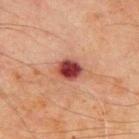Impression: No biopsy was performed on this lesion — it was imaged during a full skin examination and was not determined to be concerning. Context: The recorded lesion diameter is about 3.5 mm. A roughly 15 mm field-of-view crop from a total-body skin photograph. The patient is a male aged 68–72. The lesion is on the chest. Captured under cross-polarized illumination.